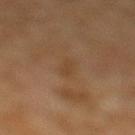Notes:
* workup — no biopsy performed (imaged during a skin exam)
* size — ≈2.5 mm
* tile lighting — cross-polarized illumination
* location — the leg
* image source — ~15 mm tile from a whole-body skin photo
* automated lesion analysis — an eccentricity of roughly 0.95 and two-axis asymmetry of about 0.4; a border-irregularity rating of about 4.5/10, a within-lesion color-variation index near 0/10, and peripheral color asymmetry of about 0
* patient — male, aged 58 to 62